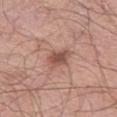The lesion was tiled from a total-body skin photograph and was not biopsied. Measured at roughly 3 mm in maximum diameter. The lesion is located on the leg. This is a white-light tile. A 15 mm close-up tile from a total-body photography series done for melanoma screening. A male subject, aged approximately 70.